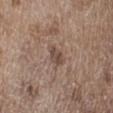Findings:
- biopsy status: no biopsy performed (imaged during a skin exam)
- subject: male, approximately 70 years of age
- size: ≈2.5 mm
- image: total-body-photography crop, ~15 mm field of view
- location: the right lower leg
- TBP lesion metrics: an average lesion color of about L≈47 a*≈16 b*≈24 (CIELAB), roughly 9 lightness units darker than nearby skin, and a lesion-to-skin contrast of about 7 (normalized; higher = more distinct); internal color variation of about 3 on a 0–10 scale and a peripheral color-asymmetry measure near 1; a detector confidence of about 100 out of 100 that the crop contains a lesion